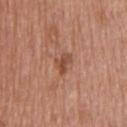Clinical impression: Recorded during total-body skin imaging; not selected for excision or biopsy. Acquisition and patient details: The total-body-photography lesion software estimated an area of roughly 4 mm² and an eccentricity of roughly 0.75. A male subject, aged 58–62. Located on the upper back. This is a white-light tile. Cropped from a total-body skin-imaging series; the visible field is about 15 mm. Measured at roughly 3 mm in maximum diameter.